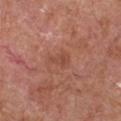Clinical impression:
Captured during whole-body skin photography for melanoma surveillance; the lesion was not biopsied.
Acquisition and patient details:
The recorded lesion diameter is about 2.5 mm. An algorithmic analysis of the crop reported an area of roughly 4 mm², an eccentricity of roughly 0.75, and a symmetry-axis asymmetry near 0.5. The software also gave an average lesion color of about L≈48 a*≈25 b*≈30 (CIELAB) and roughly 6 lightness units darker than nearby skin. The analysis additionally found a nevus-likeness score of about 0/100 and a lesion-detection confidence of about 100/100. The lesion is on the chest. The patient is a male about 65 years old. A lesion tile, about 15 mm wide, cut from a 3D total-body photograph.